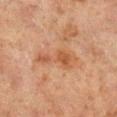biopsy_status: not biopsied; imaged during a skin examination
patient:
  sex: male
  age_approx: 65
lesion_size:
  long_diameter_mm_approx: 5.5
lighting: cross-polarized
site: left lower leg
automated_metrics:
  area_mm2_approx: 9.5
  eccentricity: 0.9
  shape_asymmetry: 0.45
  cielab_L: 44
  cielab_a: 20
  cielab_b: 30
  vs_skin_darker_L: 7.0
  lesion_detection_confidence_0_100: 100
image:
  source: total-body photography crop
  field_of_view_mm: 15